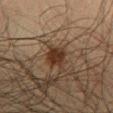Q: Was a biopsy performed?
A: no biopsy performed (imaged during a skin exam)
Q: What is the imaging modality?
A: ~15 mm crop, total-body skin-cancer survey
Q: What lighting was used for the tile?
A: cross-polarized illumination
Q: What is the anatomic site?
A: the chest
Q: Who is the patient?
A: male, approximately 30 years of age
Q: What is the lesion's diameter?
A: ≈3 mm
Q: Automated lesion metrics?
A: an automated nevus-likeness rating near 95 out of 100 and a lesion-detection confidence of about 100/100